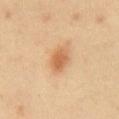Findings:
• anatomic site: the chest
• imaging modality: ~15 mm crop, total-body skin-cancer survey
• illumination: cross-polarized
• automated metrics: a footprint of about 5.5 mm², an eccentricity of roughly 0.7, and two-axis asymmetry of about 0.15
• patient: male, approximately 45 years of age